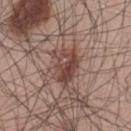Part of a total-body skin-imaging series; this lesion was reviewed on a skin check and was not flagged for biopsy.
Imaged with white-light lighting.
About 4.5 mm across.
The patient is a male roughly 70 years of age.
Located on the lower back.
Cropped from a whole-body photographic skin survey; the tile spans about 15 mm.